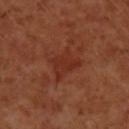workup: total-body-photography surveillance lesion; no biopsy | TBP lesion metrics: a mean CIELAB color near L≈32 a*≈28 b*≈31, a lesion–skin lightness drop of about 6, and a lesion-to-skin contrast of about 6 (normalized; higher = more distinct) | imaging modality: ~15 mm crop, total-body skin-cancer survey | location: the arm | lighting: cross-polarized | lesion size: ≈4 mm | subject: female, aged approximately 50.